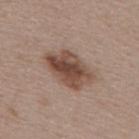Assessment: Imaged during a routine full-body skin examination; the lesion was not biopsied and no histopathology is available. Image and clinical context: A female subject, in their mid- to late 40s. The total-body-photography lesion software estimated an average lesion color of about L≈47 a*≈18 b*≈26 (CIELAB) and a normalized border contrast of about 10. The software also gave border irregularity of about 3 on a 0–10 scale, a within-lesion color-variation index near 7/10, and a peripheral color-asymmetry measure near 2.5. And it measured a classifier nevus-likeness of about 70/100. This image is a 15 mm lesion crop taken from a total-body photograph. Imaged with white-light lighting. Longest diameter approximately 5.5 mm. The lesion is located on the mid back.